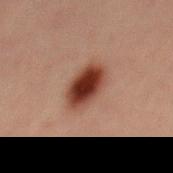Assessment: This lesion was catalogued during total-body skin photography and was not selected for biopsy. Background: A male patient roughly 30 years of age. From the mid back. A 15 mm crop from a total-body photograph taken for skin-cancer surveillance. About 5 mm across. Imaged with cross-polarized lighting.